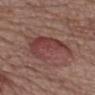biopsy status = imaged on a skin check; not biopsied
site = the left upper arm
lesion size = about 6.5 mm
patient = male, aged 73–77
image source = ~15 mm tile from a whole-body skin photo
lighting = white-light illumination